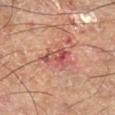Assessment: The lesion was tiled from a total-body skin photograph and was not biopsied. Background: The total-body-photography lesion software estimated a footprint of about 9.5 mm², a shape eccentricity near 0.6, and two-axis asymmetry of about 0.45. And it measured a mean CIELAB color near L≈56 a*≈30 b*≈29, a lesion–skin lightness drop of about 10, and a normalized lesion–skin contrast near 7. Cropped from a total-body skin-imaging series; the visible field is about 15 mm. From the right lower leg. This is a cross-polarized tile.